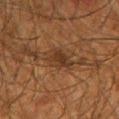The lesion was photographed on a routine skin check and not biopsied; there is no pathology result.
Located on the right forearm.
Automated image analysis of the tile measured an area of roughly 6 mm² and a shape-asymmetry score of about 0.35 (0 = symmetric). The software also gave a mean CIELAB color near L≈28 a*≈16 b*≈25, about 7 CIELAB-L* units darker than the surrounding skin, and a normalized lesion–skin contrast near 8. And it measured border irregularity of about 3.5 on a 0–10 scale, a color-variation rating of about 3.5/10, and a peripheral color-asymmetry measure near 1.
A male patient about 65 years old.
The tile uses cross-polarized illumination.
A 15 mm crop from a total-body photograph taken for skin-cancer surveillance.
Longest diameter approximately 4 mm.